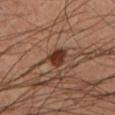<record>
<biopsy_status>not biopsied; imaged during a skin examination</biopsy_status>
<lesion_size>
  <long_diameter_mm_approx>3.0</long_diameter_mm_approx>
</lesion_size>
<site>left lower leg</site>
<patient>
  <sex>male</sex>
  <age_approx>50</age_approx>
</patient>
<image>
  <source>total-body photography crop</source>
  <field_of_view_mm>15</field_of_view_mm>
</image>
<automated_metrics>
  <area_mm2_approx>5.0</area_mm2_approx>
  <eccentricity>0.65</eccentricity>
  <shape_asymmetry>0.25</shape_asymmetry>
  <cielab_L>33</cielab_L>
  <cielab_a>21</cielab_a>
  <cielab_b>27</cielab_b>
  <color_variation_0_10>3.0</color_variation_0_10>
  <peripheral_color_asymmetry>1.0</peripheral_color_asymmetry>
  <lesion_detection_confidence_0_100>100</lesion_detection_confidence_0_100>
</automated_metrics>
<lighting>cross-polarized</lighting>
</record>